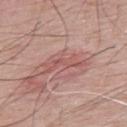This lesion was catalogued during total-body skin photography and was not selected for biopsy. On the upper back. Automated image analysis of the tile measured an average lesion color of about L≈55 a*≈25 b*≈24 (CIELAB), about 8 CIELAB-L* units darker than the surrounding skin, and a normalized lesion–skin contrast near 5.5. It also reported a nevus-likeness score of about 0/100 and a detector confidence of about 95 out of 100 that the crop contains a lesion. Captured under white-light illumination. The subject is a male roughly 65 years of age. A close-up tile cropped from a whole-body skin photograph, about 15 mm across. Longest diameter approximately 5 mm.